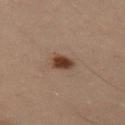Assessment: No biopsy was performed on this lesion — it was imaged during a full skin examination and was not determined to be concerning. Image and clinical context: This is a cross-polarized tile. The lesion is located on the left thigh. The lesion-visualizer software estimated a footprint of about 4.5 mm², a shape eccentricity near 0.6, and a shape-asymmetry score of about 0.15 (0 = symmetric). The software also gave an average lesion color of about L≈31 a*≈15 b*≈22 (CIELAB), about 12 CIELAB-L* units darker than the surrounding skin, and a normalized border contrast of about 11.5. It also reported border irregularity of about 1.5 on a 0–10 scale, a within-lesion color-variation index near 2.5/10, and a peripheral color-asymmetry measure near 1. It also reported a nevus-likeness score of about 100/100 and a detector confidence of about 100 out of 100 that the crop contains a lesion. A female subject, about 30 years old. A close-up tile cropped from a whole-body skin photograph, about 15 mm across. The lesion's longest dimension is about 2.5 mm.